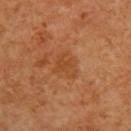biopsy status: imaged on a skin check; not biopsied
location: the upper back
image source: 15 mm crop, total-body photography
diameter: about 3 mm
lighting: cross-polarized illumination
subject: male, about 60 years old